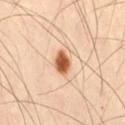Clinical impression: The lesion was photographed on a routine skin check and not biopsied; there is no pathology result. Context: A close-up tile cropped from a whole-body skin photograph, about 15 mm across. The lesion is located on the lower back. Longest diameter approximately 3 mm. Captured under cross-polarized illumination. The patient is a male roughly 40 years of age.